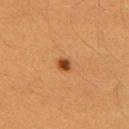Clinical impression: No biopsy was performed on this lesion — it was imaged during a full skin examination and was not determined to be concerning. Background: The total-body-photography lesion software estimated a footprint of about 2.5 mm², an eccentricity of roughly 0.55, and two-axis asymmetry of about 0.15. It also reported a lesion–skin lightness drop of about 15. The patient is a female about 40 years old. This is a cross-polarized tile. A 15 mm close-up tile from a total-body photography series done for melanoma screening. The recorded lesion diameter is about 2 mm. Located on the arm.